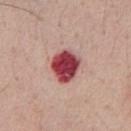biopsy status = no biopsy performed (imaged during a skin exam) | site = the front of the torso | subject = male, approximately 55 years of age | image source = total-body-photography crop, ~15 mm field of view.